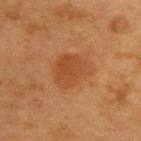A close-up tile cropped from a whole-body skin photograph, about 15 mm across.
The patient is a female aged 38–42.
The lesion is located on the upper back.